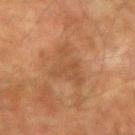Findings:
• follow-up · catalogued during a skin exam; not biopsied
• TBP lesion metrics · a footprint of about 10 mm², a shape eccentricity near 0.75, and a symmetry-axis asymmetry near 0.55; a lesion color around L≈42 a*≈19 b*≈31 in CIELAB, a lesion–skin lightness drop of about 6, and a normalized border contrast of about 5.5; a classifier nevus-likeness of about 0/100 and lesion-presence confidence of about 100/100
• patient · male, in their mid-70s
• size · ~5 mm (longest diameter)
• illumination · cross-polarized illumination
• image · ~15 mm crop, total-body skin-cancer survey
• site · the right upper arm